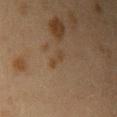Part of a total-body skin-imaging series; this lesion was reviewed on a skin check and was not flagged for biopsy.